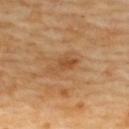Q: Was a biopsy performed?
A: no biopsy performed (imaged during a skin exam)
Q: Who is the patient?
A: female, aged 58 to 62
Q: What is the lesion's diameter?
A: ≈3.5 mm
Q: What kind of image is this?
A: ~15 mm tile from a whole-body skin photo
Q: How was the tile lit?
A: cross-polarized illumination
Q: Where on the body is the lesion?
A: the back